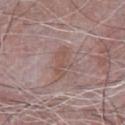Findings:
• tile lighting — white-light
• image — ~15 mm crop, total-body skin-cancer survey
• size — about 4 mm
• location — the front of the torso
• subject — male, roughly 65 years of age